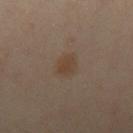Q: Where on the body is the lesion?
A: the leg
Q: Patient demographics?
A: female, aged 33 to 37
Q: What is the imaging modality?
A: ~15 mm tile from a whole-body skin photo